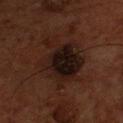Case summary:
– biopsy status — imaged on a skin check; not biopsied
– illumination — cross-polarized
– diameter — about 6 mm
– TBP lesion metrics — a footprint of about 24 mm², an outline eccentricity of about 0.3 (0 = round, 1 = elongated), and two-axis asymmetry of about 0.3; a lesion color around L≈14 a*≈14 b*≈16 in CIELAB, a lesion–skin lightness drop of about 8, and a normalized lesion–skin contrast near 12
– anatomic site — the front of the torso
– imaging modality — total-body-photography crop, ~15 mm field of view
– subject — male, aged approximately 55